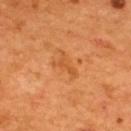Captured during whole-body skin photography for melanoma surveillance; the lesion was not biopsied.
Cropped from a whole-body photographic skin survey; the tile spans about 15 mm.
The total-body-photography lesion software estimated a mean CIELAB color near L≈50 a*≈27 b*≈43, a lesion–skin lightness drop of about 6, and a lesion-to-skin contrast of about 5 (normalized; higher = more distinct). The software also gave a border-irregularity rating of about 6.5/10 and peripheral color asymmetry of about 0. It also reported a classifier nevus-likeness of about 0/100.
A male patient approximately 55 years of age.
Located on the upper back.
The tile uses cross-polarized illumination.